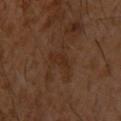<case>
  <biopsy_status>not biopsied; imaged during a skin examination</biopsy_status>
  <lesion_size>
    <long_diameter_mm_approx>3.0</long_diameter_mm_approx>
  </lesion_size>
  <patient>
    <sex>male</sex>
    <age_approx>30</age_approx>
  </patient>
  <lighting>cross-polarized</lighting>
  <automated_metrics>
    <border_irregularity_0_10>3.5</border_irregularity_0_10>
    <color_variation_0_10>0.5</color_variation_0_10>
  </automated_metrics>
  <image>
    <source>total-body photography crop</source>
    <field_of_view_mm>15</field_of_view_mm>
  </image>
  <site>upper back</site>
</case>